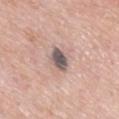biopsy status = total-body-photography surveillance lesion; no biopsy
anatomic site = the mid back
image source = 15 mm crop, total-body photography
illumination = white-light
size = ~3.5 mm (longest diameter)
subject = male, aged 53–57